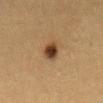Notes:
• notes — imaged on a skin check; not biopsied
• subject — female, aged 38 to 42
• diameter — ~3 mm (longest diameter)
• tile lighting — cross-polarized
• automated metrics — a footprint of about 4.5 mm², an eccentricity of roughly 0.65, and a shape-asymmetry score of about 0.2 (0 = symmetric); a lesion color around L≈33 a*≈16 b*≈28 in CIELAB and a normalized border contrast of about 12.5; border irregularity of about 1.5 on a 0–10 scale, internal color variation of about 5 on a 0–10 scale, and peripheral color asymmetry of about 1.5
• location — the mid back
• imaging modality — total-body-photography crop, ~15 mm field of view